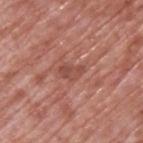Impression:
The lesion was photographed on a routine skin check and not biopsied; there is no pathology result.
Clinical summary:
The lesion's longest dimension is about 3 mm. A close-up tile cropped from a whole-body skin photograph, about 15 mm across. The tile uses white-light illumination. On the upper back. The subject is a male approximately 70 years of age.